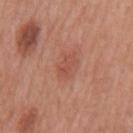Findings:
* notes · imaged on a skin check; not biopsied
* location · the mid back
* lighting · white-light
* image source · ~15 mm tile from a whole-body skin photo
* lesion size · ~3 mm (longest diameter)
* TBP lesion metrics · two-axis asymmetry of about 0.25; a border-irregularity index near 2.5/10 and a within-lesion color-variation index near 2.5/10; a classifier nevus-likeness of about 15/100 and a detector confidence of about 100 out of 100 that the crop contains a lesion
* patient · male, aged 68–72